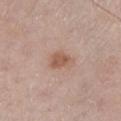Impression:
Part of a total-body skin-imaging series; this lesion was reviewed on a skin check and was not flagged for biopsy.
Background:
About 3.5 mm across. Automated image analysis of the tile measured an average lesion color of about L≈57 a*≈19 b*≈28 (CIELAB) and roughly 9 lightness units darker than nearby skin. The analysis additionally found a lesion-detection confidence of about 100/100. A 15 mm close-up extracted from a 3D total-body photography capture. Imaged with white-light lighting. On the left lower leg. The patient is a male about 60 years old.